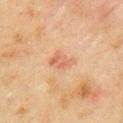Assessment:
The lesion was tiled from a total-body skin photograph and was not biopsied.
Context:
The subject is a male aged approximately 45. On the left upper arm. A 15 mm close-up extracted from a 3D total-body photography capture.